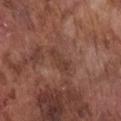Q: Was a biopsy performed?
A: no biopsy performed (imaged during a skin exam)
Q: What did automated image analysis measure?
A: an area of roughly 3 mm², an outline eccentricity of about 0.95 (0 = round, 1 = elongated), and two-axis asymmetry of about 0.45; an average lesion color of about L≈38 a*≈21 b*≈25 (CIELAB), about 7 CIELAB-L* units darker than the surrounding skin, and a normalized lesion–skin contrast near 6; a border-irregularity index near 5.5/10, a within-lesion color-variation index near 0/10, and a peripheral color-asymmetry measure near 0
Q: What is the imaging modality?
A: ~15 mm crop, total-body skin-cancer survey
Q: What is the lesion's diameter?
A: about 3 mm
Q: What are the patient's age and sex?
A: male, aged 73–77
Q: Where on the body is the lesion?
A: the front of the torso